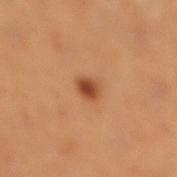follow-up: total-body-photography surveillance lesion; no biopsy | illumination: cross-polarized | lesion size: ≈2 mm | subject: female, about 55 years old | location: the right lower leg | acquisition: total-body-photography crop, ~15 mm field of view.